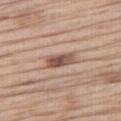diameter: about 4 mm; site: the right thigh; image: ~15 mm tile from a whole-body skin photo; patient: male, aged 68 to 72; tile lighting: white-light.